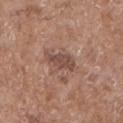This lesion was catalogued during total-body skin photography and was not selected for biopsy. Cropped from a total-body skin-imaging series; the visible field is about 15 mm. Located on the arm. The subject is a male about 70 years old.